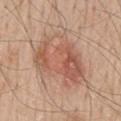Assessment: The lesion was photographed on a routine skin check and not biopsied; there is no pathology result. Clinical summary: The recorded lesion diameter is about 7.5 mm. The lesion is on the mid back. The total-body-photography lesion software estimated border irregularity of about 3.5 on a 0–10 scale, a color-variation rating of about 7/10, and a peripheral color-asymmetry measure near 2.5. The subject is a male in their 60s. Captured under white-light illumination. A close-up tile cropped from a whole-body skin photograph, about 15 mm across.